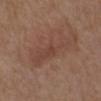{"site": "upper back", "lighting": "white-light", "patient": {"sex": "male", "age_approx": 70}, "image": {"source": "total-body photography crop", "field_of_view_mm": 15}, "lesion_size": {"long_diameter_mm_approx": 1.5}}